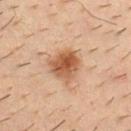biopsy_status: not biopsied; imaged during a skin examination
image:
  source: total-body photography crop
  field_of_view_mm: 15
patient:
  sex: male
  age_approx: 35
site: chest